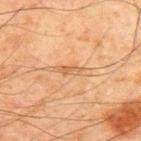Clinical impression: No biopsy was performed on this lesion — it was imaged during a full skin examination and was not determined to be concerning. Image and clinical context: The tile uses cross-polarized illumination. The subject is a male aged 68–72. About 2.5 mm across. A roughly 15 mm field-of-view crop from a total-body skin photograph. Located on the chest.